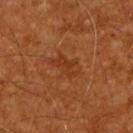workup: catalogued during a skin exam; not biopsied
image: ~15 mm crop, total-body skin-cancer survey
automated lesion analysis: an eccentricity of roughly 0.85 and a shape-asymmetry score of about 0.3 (0 = symmetric); a mean CIELAB color near L≈33 a*≈24 b*≈34 and a normalized border contrast of about 5; internal color variation of about 2.5 on a 0–10 scale and a peripheral color-asymmetry measure near 1
illumination: cross-polarized
patient: male, aged around 60
location: the upper back
lesion diameter: ≈3.5 mm